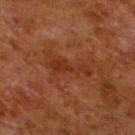Approximately 5 mm at its widest.
From the left lower leg.
The total-body-photography lesion software estimated a lesion area of about 6.5 mm², an outline eccentricity of about 0.95 (0 = round, 1 = elongated), and a symmetry-axis asymmetry near 0.55. The software also gave an average lesion color of about L≈25 a*≈21 b*≈27 (CIELAB) and a normalized lesion–skin contrast near 6.
Captured under cross-polarized illumination.
Cropped from a whole-body photographic skin survey; the tile spans about 15 mm.
A male subject, aged 78–82.